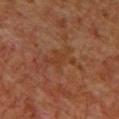Notes:
– follow-up: total-body-photography surveillance lesion; no biopsy
– site: the front of the torso
– subject: male, approximately 60 years of age
– acquisition: 15 mm crop, total-body photography